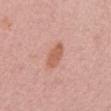Impression:
The lesion was photographed on a routine skin check and not biopsied; there is no pathology result.
Context:
The lesion is on the chest. A 15 mm close-up tile from a total-body photography series done for melanoma screening. A female subject aged 28–32.